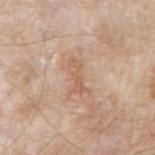Imaged during a routine full-body skin examination; the lesion was not biopsied and no histopathology is available.
This image is a 15 mm lesion crop taken from a total-body photograph.
A male subject aged around 80.
From the right upper arm.